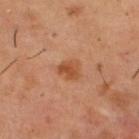The lesion was photographed on a routine skin check and not biopsied; there is no pathology result. A male patient in their mid- to late 50s. The lesion is on the upper back. A 15 mm close-up tile from a total-body photography series done for melanoma screening.